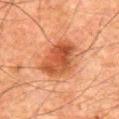Clinical impression: The lesion was photographed on a routine skin check and not biopsied; there is no pathology result. Acquisition and patient details: The patient is a male aged around 80. Cropped from a whole-body photographic skin survey; the tile spans about 15 mm. On the abdomen.